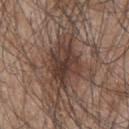<record>
<biopsy_status>not biopsied; imaged during a skin examination</biopsy_status>
<image>
  <source>total-body photography crop</source>
  <field_of_view_mm>15</field_of_view_mm>
</image>
<site>upper back</site>
<patient>
  <sex>male</sex>
  <age_approx>45</age_approx>
</patient>
</record>